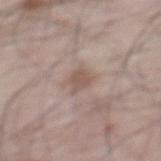{
  "biopsy_status": "not biopsied; imaged during a skin examination",
  "site": "back",
  "patient": {
    "sex": "male",
    "age_approx": 65
  },
  "lesion_size": {
    "long_diameter_mm_approx": 2.5
  },
  "image": {
    "source": "total-body photography crop",
    "field_of_view_mm": 15
  },
  "lighting": "white-light",
  "automated_metrics": {
    "cielab_L": 54,
    "cielab_a": 15,
    "cielab_b": 24,
    "vs_skin_darker_L": 9.0,
    "vs_skin_contrast_norm": 6.5,
    "border_irregularity_0_10": 3.0,
    "color_variation_0_10": 0.5,
    "peripheral_color_asymmetry": 0.0
  }
}